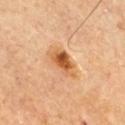The lesion was tiled from a total-body skin photograph and was not biopsied.
The total-body-photography lesion software estimated a footprint of about 7.5 mm² and an outline eccentricity of about 0.85 (0 = round, 1 = elongated). And it measured a lesion color around L≈57 a*≈25 b*≈41 in CIELAB and a normalized border contrast of about 9.
Located on the chest.
Cropped from a total-body skin-imaging series; the visible field is about 15 mm.
Measured at roughly 4.5 mm in maximum diameter.
A male subject, in their mid-80s or older.
The tile uses cross-polarized illumination.